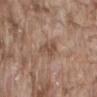Findings:
• workup: catalogued during a skin exam; not biopsied
• acquisition: 15 mm crop, total-body photography
• anatomic site: the lower back
• tile lighting: white-light illumination
• patient: male, aged approximately 75
• lesion size: ~3.5 mm (longest diameter)
• image-analysis metrics: a footprint of about 5.5 mm², an outline eccentricity of about 0.85 (0 = round, 1 = elongated), and a symmetry-axis asymmetry near 0.3; a lesion color around L≈50 a*≈17 b*≈27 in CIELAB; a border-irregularity rating of about 3/10, a within-lesion color-variation index near 2/10, and radial color variation of about 1